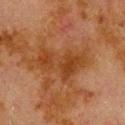The lesion was photographed on a routine skin check and not biopsied; there is no pathology result.
Imaged with cross-polarized lighting.
The subject is a male in their 80s.
On the upper back.
About 6.5 mm across.
An algorithmic analysis of the crop reported a footprint of about 14 mm², an outline eccentricity of about 0.85 (0 = round, 1 = elongated), and a shape-asymmetry score of about 0.45 (0 = symmetric). And it measured roughly 7 lightness units darker than nearby skin and a lesion-to-skin contrast of about 7 (normalized; higher = more distinct). The software also gave an automated nevus-likeness rating near 0 out of 100 and lesion-presence confidence of about 100/100.
This image is a 15 mm lesion crop taken from a total-body photograph.